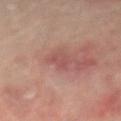Clinical impression:
This lesion was catalogued during total-body skin photography and was not selected for biopsy.
Context:
Measured at roughly 3 mm in maximum diameter. From the left forearm. A male subject, aged 58 to 62. This is a cross-polarized tile. A region of skin cropped from a whole-body photographic capture, roughly 15 mm wide.